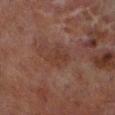biopsy_status: not biopsied; imaged during a skin examination
lighting: cross-polarized
site: left lower leg
patient:
  sex: male
  age_approx: 70
image:
  source: total-body photography crop
  field_of_view_mm: 15